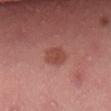No biopsy was performed on this lesion — it was imaged during a full skin examination and was not determined to be concerning. A roughly 15 mm field-of-view crop from a total-body skin photograph. Longest diameter approximately 3 mm. From the lower back. Captured under white-light illumination. The patient is a male aged approximately 40.